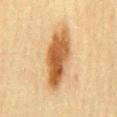Q: Is there a histopathology result?
A: imaged on a skin check; not biopsied
Q: What kind of image is this?
A: total-body-photography crop, ~15 mm field of view
Q: Who is the patient?
A: female, in their mid- to late 50s
Q: Where on the body is the lesion?
A: the mid back